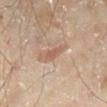Notes:
* notes: total-body-photography surveillance lesion; no biopsy
* subject: male, aged 43–47
* image source: 15 mm crop, total-body photography
* size: ≈3 mm
* location: the left lower leg
* lighting: cross-polarized illumination